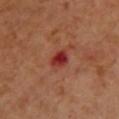Q: Was a biopsy performed?
A: total-body-photography surveillance lesion; no biopsy
Q: How was the tile lit?
A: cross-polarized illumination
Q: What is the lesion's diameter?
A: ~2.5 mm (longest diameter)
Q: What are the patient's age and sex?
A: female, aged approximately 55
Q: What did automated image analysis measure?
A: a footprint of about 4.5 mm² and a symmetry-axis asymmetry near 0.25; a lesion color around L≈37 a*≈36 b*≈30 in CIELAB and a lesion-to-skin contrast of about 9.5 (normalized; higher = more distinct); a color-variation rating of about 4/10 and peripheral color asymmetry of about 1; a nevus-likeness score of about 0/100 and a detector confidence of about 100 out of 100 that the crop contains a lesion
Q: Where on the body is the lesion?
A: the chest
Q: What kind of image is this?
A: ~15 mm crop, total-body skin-cancer survey